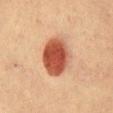The lesion was tiled from a total-body skin photograph and was not biopsied. The lesion is on the abdomen. A female patient, aged around 40. A lesion tile, about 15 mm wide, cut from a 3D total-body photograph. The tile uses cross-polarized illumination. Measured at roughly 5.5 mm in maximum diameter. An algorithmic analysis of the crop reported an eccentricity of roughly 0.65 and two-axis asymmetry of about 0.15. The software also gave a lesion color around L≈45 a*≈26 b*≈31 in CIELAB and a normalized lesion–skin contrast near 12. And it measured a classifier nevus-likeness of about 100/100.